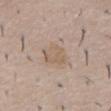No biopsy was performed on this lesion — it was imaged during a full skin examination and was not determined to be concerning. A male subject in their 50s. Approximately 3 mm at its widest. On the chest. A roughly 15 mm field-of-view crop from a total-body skin photograph.